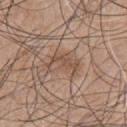biopsy status: total-body-photography surveillance lesion; no biopsy
patient: male, in their mid- to late 40s
size: ~4 mm (longest diameter)
lighting: white-light illumination
image: ~15 mm crop, total-body skin-cancer survey
body site: the front of the torso